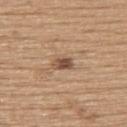follow-up = no biopsy performed (imaged during a skin exam); patient = male, approximately 65 years of age; illumination = white-light illumination; body site = the upper back; lesion diameter = ≈2.5 mm; image = ~15 mm crop, total-body skin-cancer survey.